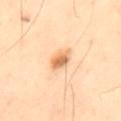Recorded during total-body skin imaging; not selected for excision or biopsy. A male subject aged 53–57. The lesion is located on the back. Cropped from a whole-body photographic skin survey; the tile spans about 15 mm.